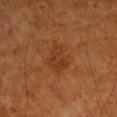The lesion was photographed on a routine skin check and not biopsied; there is no pathology result. The total-body-photography lesion software estimated an average lesion color of about L≈39 a*≈27 b*≈38 (CIELAB) and a normalized lesion–skin contrast near 5.5. And it measured an automated nevus-likeness rating near 10 out of 100. About 4 mm across. The lesion is on the left forearm. A lesion tile, about 15 mm wide, cut from a 3D total-body photograph. The tile uses cross-polarized illumination. A female patient approximately 70 years of age.